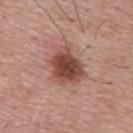Q: Is there a histopathology result?
A: total-body-photography surveillance lesion; no biopsy
Q: What kind of image is this?
A: total-body-photography crop, ~15 mm field of view
Q: What is the lesion's diameter?
A: ~5 mm (longest diameter)
Q: How was the tile lit?
A: white-light
Q: What are the patient's age and sex?
A: male, approximately 55 years of age